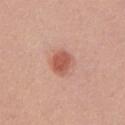No biopsy was performed on this lesion — it was imaged during a full skin examination and was not determined to be concerning.
A female patient about 55 years old.
Approximately 3.5 mm at its widest.
A region of skin cropped from a whole-body photographic capture, roughly 15 mm wide.
On the front of the torso.
The tile uses white-light illumination.